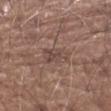<case>
<biopsy_status>not biopsied; imaged during a skin examination</biopsy_status>
<patient>
  <sex>male</sex>
  <age_approx>75</age_approx>
</patient>
<lighting>white-light</lighting>
<site>left forearm</site>
<automated_metrics>
  <nevus_likeness_0_100>0</nevus_likeness_0_100>
  <lesion_detection_confidence_0_100>80</lesion_detection_confidence_0_100>
</automated_metrics>
<lesion_size>
  <long_diameter_mm_approx>3.5</long_diameter_mm_approx>
</lesion_size>
<image>
  <source>total-body photography crop</source>
  <field_of_view_mm>15</field_of_view_mm>
</image>
</case>